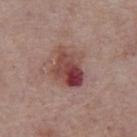notes=total-body-photography surveillance lesion; no biopsy | acquisition=15 mm crop, total-body photography | illumination=white-light | location=the chest | lesion size=~5 mm (longest diameter) | patient=male, aged 73 to 77.